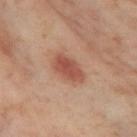Q: Was this lesion biopsied?
A: total-body-photography surveillance lesion; no biopsy
Q: What kind of image is this?
A: total-body-photography crop, ~15 mm field of view
Q: Where on the body is the lesion?
A: the left thigh
Q: Who is the patient?
A: female, aged 53 to 57
Q: Lesion size?
A: ≈4 mm